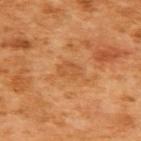workup: catalogued during a skin exam; not biopsied | lesion diameter: about 3 mm | location: the upper back | imaging modality: ~15 mm tile from a whole-body skin photo | illumination: cross-polarized | subject: female, in their mid-50s.